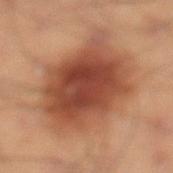workup: imaged on a skin check; not biopsied
image source: 15 mm crop, total-body photography
lesion size: ~8.5 mm (longest diameter)
site: the left lower leg
patient: male, aged 38 to 42
illumination: cross-polarized illumination